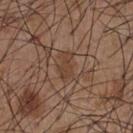biopsy status: catalogued during a skin exam; not biopsied | subject: male, in their mid- to late 50s | illumination: white-light illumination | diameter: ~3 mm (longest diameter) | image-analysis metrics: a lesion–skin lightness drop of about 6 and a normalized border contrast of about 5.5; a classifier nevus-likeness of about 10/100 and a lesion-detection confidence of about 85/100 | image source: ~15 mm crop, total-body skin-cancer survey | anatomic site: the chest.